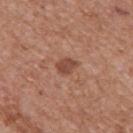Clinical impression:
Captured during whole-body skin photography for melanoma surveillance; the lesion was not biopsied.
Image and clinical context:
A 15 mm close-up extracted from a 3D total-body photography capture. The lesion-visualizer software estimated a lesion color around L≈46 a*≈24 b*≈29 in CIELAB and about 10 CIELAB-L* units darker than the surrounding skin. Captured under white-light illumination. On the back. The subject is a male in their mid- to late 60s. Approximately 2.5 mm at its widest.